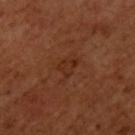Notes:
* biopsy status · no biopsy performed (imaged during a skin exam)
* TBP lesion metrics · a nevus-likeness score of about 0/100 and lesion-presence confidence of about 100/100
* image source · total-body-photography crop, ~15 mm field of view
* size · ~3.5 mm (longest diameter)
* subject · male, aged around 50
* illumination · cross-polarized
* body site · the upper back